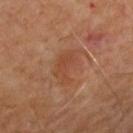* notes — imaged on a skin check; not biopsied
* lighting — cross-polarized
* body site — the upper back
* diameter — ≈5 mm
* acquisition — ~15 mm tile from a whole-body skin photo
* subject — male, in their mid- to late 60s